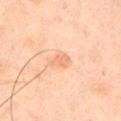Captured during whole-body skin photography for melanoma surveillance; the lesion was not biopsied.
The lesion is on the chest.
This is a cross-polarized tile.
A lesion tile, about 15 mm wide, cut from a 3D total-body photograph.
A male patient roughly 45 years of age.